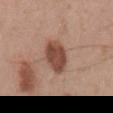| field | value |
|---|---|
| notes | total-body-photography surveillance lesion; no biopsy |
| patient | male, aged approximately 50 |
| image source | 15 mm crop, total-body photography |
| lesion diameter | about 4.5 mm |
| lighting | white-light illumination |
| site | the mid back |
| automated lesion analysis | a footprint of about 10 mm² and two-axis asymmetry of about 0.2 |